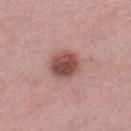No biopsy was performed on this lesion — it was imaged during a full skin examination and was not determined to be concerning. Automated image analysis of the tile measured an average lesion color of about L≈49 a*≈23 b*≈24 (CIELAB), a lesion–skin lightness drop of about 14, and a lesion-to-skin contrast of about 10 (normalized; higher = more distinct). And it measured an automated nevus-likeness rating near 90 out of 100 and lesion-presence confidence of about 100/100. This image is a 15 mm lesion crop taken from a total-body photograph. The tile uses white-light illumination. The subject is a female about 40 years old. The lesion is on the left lower leg. About 4 mm across.